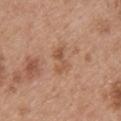Q: Automated lesion metrics?
A: a lesion area of about 5 mm², an outline eccentricity of about 0.8 (0 = round, 1 = elongated), and a shape-asymmetry score of about 0.45 (0 = symmetric); an average lesion color of about L≈54 a*≈21 b*≈32 (CIELAB) and a lesion–skin lightness drop of about 8
Q: Where on the body is the lesion?
A: the upper back
Q: How was this image acquired?
A: ~15 mm crop, total-body skin-cancer survey
Q: Who is the patient?
A: male, about 50 years old
Q: Lesion size?
A: about 3.5 mm